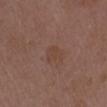Assessment: Captured during whole-body skin photography for melanoma surveillance; the lesion was not biopsied. Background: Imaged with white-light lighting. On the left upper arm. The patient is a female in their 30s. A 15 mm close-up tile from a total-body photography series done for melanoma screening. The lesion's longest dimension is about 2.5 mm. An algorithmic analysis of the crop reported a lesion area of about 4 mm² and an outline eccentricity of about 0.6 (0 = round, 1 = elongated). The analysis additionally found a lesion color around L≈42 a*≈18 b*≈26 in CIELAB, a lesion–skin lightness drop of about 4, and a normalized border contrast of about 4.5. The software also gave a nevus-likeness score of about 0/100.